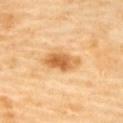The lesion was tiled from a total-body skin photograph and was not biopsied. Longest diameter approximately 5 mm. The lesion is on the back. A female patient, aged 58 to 62. Imaged with cross-polarized lighting. An algorithmic analysis of the crop reported an area of roughly 9 mm², an outline eccentricity of about 0.9 (0 = round, 1 = elongated), and a shape-asymmetry score of about 0.2 (0 = symmetric). The software also gave radial color variation of about 2. The software also gave a classifier nevus-likeness of about 95/100 and a detector confidence of about 100 out of 100 that the crop contains a lesion. Cropped from a total-body skin-imaging series; the visible field is about 15 mm.